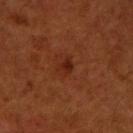Notes:
– tile lighting — cross-polarized illumination
– lesion size — ~2 mm (longest diameter)
– automated lesion analysis — an area of roughly 2.5 mm², an outline eccentricity of about 0.65 (0 = round, 1 = elongated), and two-axis asymmetry of about 0.3; a lesion–skin lightness drop of about 7 and a normalized border contrast of about 7.5; an automated nevus-likeness rating near 60 out of 100 and a lesion-detection confidence of about 100/100
– imaging modality — ~15 mm tile from a whole-body skin photo
– location — the right upper arm
– patient — male, in their mid- to late 40s